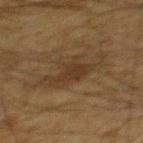notes = total-body-photography surveillance lesion; no biopsy
acquisition = ~15 mm tile from a whole-body skin photo
patient = male, in their 60s
illumination = cross-polarized
site = the upper back